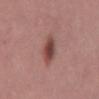Impression: The lesion was photographed on a routine skin check and not biopsied; there is no pathology result. Context: The lesion's longest dimension is about 4 mm. Captured under white-light illumination. Cropped from a total-body skin-imaging series; the visible field is about 15 mm. The patient is a male aged 38 to 42. The lesion is on the mid back.